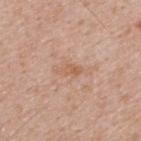Case summary:
• notes · no biopsy performed (imaged during a skin exam)
• imaging modality · total-body-photography crop, ~15 mm field of view
• diameter · ~3 mm (longest diameter)
• subject · male, in their 40s
• site · the upper back